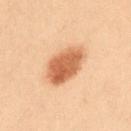Case summary:
– follow-up · catalogued during a skin exam; not biopsied
– image source · 15 mm crop, total-body photography
– lighting · cross-polarized illumination
– image-analysis metrics · an area of roughly 14 mm², an outline eccentricity of about 0.75 (0 = round, 1 = elongated), and a symmetry-axis asymmetry near 0.15; an average lesion color of about L≈50 a*≈21 b*≈33 (CIELAB), roughly 13 lightness units darker than nearby skin, and a lesion-to-skin contrast of about 9.5 (normalized; higher = more distinct); a classifier nevus-likeness of about 100/100 and a lesion-detection confidence of about 100/100
– anatomic site · the abdomen
– patient · female, in their 40s
– size · ≈5 mm